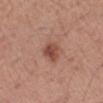{
  "biopsy_status": "not biopsied; imaged during a skin examination",
  "lesion_size": {
    "long_diameter_mm_approx": 2.5
  },
  "automated_metrics": {
    "area_mm2_approx": 4.5,
    "eccentricity": 0.5,
    "shape_asymmetry": 0.25
  },
  "patient": {
    "sex": "male",
    "age_approx": 60
  },
  "lighting": "white-light",
  "image": {
    "source": "total-body photography crop",
    "field_of_view_mm": 15
  },
  "site": "arm"
}